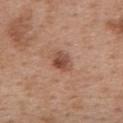- illumination: white-light illumination
- anatomic site: the back
- imaging modality: ~15 mm tile from a whole-body skin photo
- size: ≈3 mm
- TBP lesion metrics: an area of roughly 5 mm², an outline eccentricity of about 0.6 (0 = round, 1 = elongated), and two-axis asymmetry of about 0.2; a mean CIELAB color near L≈49 a*≈23 b*≈29 and a normalized border contrast of about 8
- patient: female, aged approximately 40